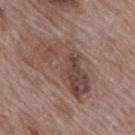<record>
  <biopsy_status>not biopsied; imaged during a skin examination</biopsy_status>
  <image>
    <source>total-body photography crop</source>
    <field_of_view_mm>15</field_of_view_mm>
  </image>
  <patient>
    <sex>male</sex>
    <age_approx>70</age_approx>
  </patient>
  <site>back</site>
  <lesion_size>
    <long_diameter_mm_approx>8.0</long_diameter_mm_approx>
  </lesion_size>
  <lighting>white-light</lighting>
</record>